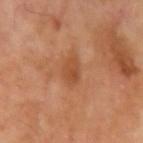Impression: No biopsy was performed on this lesion — it was imaged during a full skin examination and was not determined to be concerning. Clinical summary: Approximately 3 mm at its widest. The subject is a male roughly 70 years of age. A close-up tile cropped from a whole-body skin photograph, about 15 mm across. The lesion is on the left upper arm.